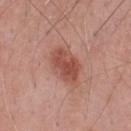follow-up: no biopsy performed (imaged during a skin exam) | site: the front of the torso | patient: male, aged approximately 60 | size: ≈4.5 mm | TBP lesion metrics: a nevus-likeness score of about 95/100 | imaging modality: ~15 mm tile from a whole-body skin photo | tile lighting: white-light.